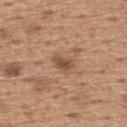  biopsy_status: not biopsied; imaged during a skin examination
  lighting: white-light
  image:
    source: total-body photography crop
    field_of_view_mm: 15
  automated_metrics:
    area_mm2_approx: 3.5
    eccentricity: 0.8
    shape_asymmetry: 0.25
    vs_skin_darker_L: 11.0
    vs_skin_contrast_norm: 8.0
    border_irregularity_0_10: 2.5
    color_variation_0_10: 1.5
    peripheral_color_asymmetry: 0.5
    nevus_likeness_0_100: 25
    lesion_detection_confidence_0_100: 100
  lesion_size:
    long_diameter_mm_approx: 3.0
  patient:
    sex: male
    age_approx: 65
  site: upper back